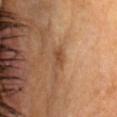Clinical impression:
This lesion was catalogued during total-body skin photography and was not selected for biopsy.
Background:
The tile uses cross-polarized illumination. A male subject, approximately 60 years of age. The lesion's longest dimension is about 2.5 mm. An algorithmic analysis of the crop reported an area of roughly 3.5 mm², an eccentricity of roughly 0.85, and two-axis asymmetry of about 0.35. It also reported a lesion-detection confidence of about 100/100. The lesion is located on the head or neck. Cropped from a total-body skin-imaging series; the visible field is about 15 mm.